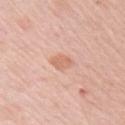The lesion was photographed on a routine skin check and not biopsied; there is no pathology result. Cropped from a whole-body photographic skin survey; the tile spans about 15 mm. This is a white-light tile. The recorded lesion diameter is about 2.5 mm. The subject is a female roughly 65 years of age. On the right upper arm. The total-body-photography lesion software estimated a lesion color around L≈66 a*≈23 b*≈31 in CIELAB, roughly 8 lightness units darker than nearby skin, and a normalized lesion–skin contrast near 5.5. It also reported border irregularity of about 2 on a 0–10 scale, a color-variation rating of about 1.5/10, and a peripheral color-asymmetry measure near 0.5. The software also gave an automated nevus-likeness rating near 10 out of 100 and a detector confidence of about 100 out of 100 that the crop contains a lesion.